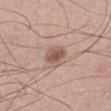This lesion was catalogued during total-body skin photography and was not selected for biopsy. Cropped from a whole-body photographic skin survey; the tile spans about 15 mm. Located on the left lower leg. A male subject, aged approximately 35.